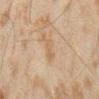Background:
About 3 mm across. Cropped from a whole-body photographic skin survey; the tile spans about 15 mm. From the right forearm. The lesion-visualizer software estimated a border-irregularity index near 4/10, a within-lesion color-variation index near 0.5/10, and peripheral color asymmetry of about 0. The software also gave a nevus-likeness score of about 0/100 and lesion-presence confidence of about 100/100. Imaged with cross-polarized lighting. A male subject aged 43–47.